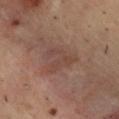Clinical impression: Recorded during total-body skin imaging; not selected for excision or biopsy. Acquisition and patient details: Automated tile analysis of the lesion measured an area of roughly 9 mm², an eccentricity of roughly 0.65, and a shape-asymmetry score of about 0.55 (0 = symmetric). And it measured a classifier nevus-likeness of about 0/100 and lesion-presence confidence of about 100/100. Imaged with cross-polarized lighting. From the back. The lesion's longest dimension is about 4.5 mm. A roughly 15 mm field-of-view crop from a total-body skin photograph. A male subject aged 53–57.